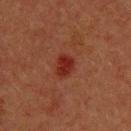biopsy_status: not biopsied; imaged during a skin examination
image:
  source: total-body photography crop
  field_of_view_mm: 15
lighting: cross-polarized
site: upper back
patient:
  sex: male
  age_approx: 40